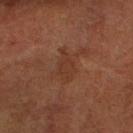Captured during whole-body skin photography for melanoma surveillance; the lesion was not biopsied.
The lesion is on the right forearm.
About 3.5 mm across.
Automated image analysis of the tile measured border irregularity of about 4 on a 0–10 scale, a color-variation rating of about 1.5/10, and a peripheral color-asymmetry measure near 0.5. It also reported a detector confidence of about 100 out of 100 that the crop contains a lesion.
A male subject about 75 years old.
The tile uses cross-polarized illumination.
A roughly 15 mm field-of-view crop from a total-body skin photograph.